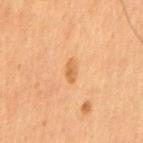Captured during whole-body skin photography for melanoma surveillance; the lesion was not biopsied.
The patient is a male in their mid- to late 50s.
Located on the abdomen.
The lesion's longest dimension is about 2.5 mm.
The total-body-photography lesion software estimated an average lesion color of about L≈59 a*≈22 b*≈42 (CIELAB), a lesion–skin lightness drop of about 8, and a normalized border contrast of about 6.5.
A 15 mm crop from a total-body photograph taken for skin-cancer surveillance.